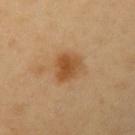Located on the left upper arm. Cropped from a whole-body photographic skin survey; the tile spans about 15 mm. The patient is a male roughly 40 years of age.